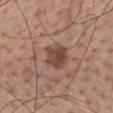automated lesion analysis = an automated nevus-likeness rating near 85 out of 100 and a lesion-detection confidence of about 100/100
acquisition = 15 mm crop, total-body photography
illumination = white-light illumination
subject = male, aged 68–72
site = the back